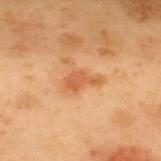| key | value |
|---|---|
| workup | imaged on a skin check; not biopsied |
| image-analysis metrics | a shape eccentricity near 0.85 and a symmetry-axis asymmetry near 0.45; a mean CIELAB color near L≈48 a*≈23 b*≈35 and about 8 CIELAB-L* units darker than the surrounding skin; an automated nevus-likeness rating near 5 out of 100 and a detector confidence of about 100 out of 100 that the crop contains a lesion |
| anatomic site | the upper back |
| imaging modality | 15 mm crop, total-body photography |
| subject | male, about 55 years old |
| size | ≈3.5 mm |